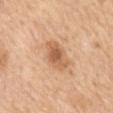Q: Is there a histopathology result?
A: catalogued during a skin exam; not biopsied
Q: How was this image acquired?
A: total-body-photography crop, ~15 mm field of view
Q: How large is the lesion?
A: about 4 mm
Q: Who is the patient?
A: female, in their mid- to late 60s
Q: Where on the body is the lesion?
A: the mid back